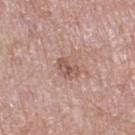This lesion was catalogued during total-body skin photography and was not selected for biopsy. The lesion is located on the right thigh. Cropped from a total-body skin-imaging series; the visible field is about 15 mm. Imaged with white-light lighting. A female subject, aged around 75. Measured at roughly 3 mm in maximum diameter. An algorithmic analysis of the crop reported an area of roughly 3.5 mm², a shape eccentricity near 0.8, and a symmetry-axis asymmetry near 0.4. The software also gave border irregularity of about 4.5 on a 0–10 scale and radial color variation of about 0.5. And it measured an automated nevus-likeness rating near 0 out of 100 and a detector confidence of about 100 out of 100 that the crop contains a lesion.